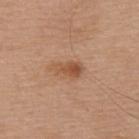The lesion was photographed on a routine skin check and not biopsied; there is no pathology result. A 15 mm close-up extracted from a 3D total-body photography capture. Approximately 3.5 mm at its widest. Located on the upper back. A male subject, aged approximately 65. The tile uses white-light illumination.